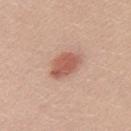patient=male, aged 28–32
imaging modality=15 mm crop, total-body photography
illumination=white-light illumination